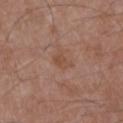image=~15 mm tile from a whole-body skin photo | patient=male, aged approximately 50 | size=about 2.5 mm | illumination=white-light | site=the left upper arm.